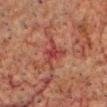| key | value |
|---|---|
| notes | no biopsy performed (imaged during a skin exam) |
| site | the head or neck |
| patient | male, aged approximately 60 |
| acquisition | 15 mm crop, total-body photography |
| TBP lesion metrics | border irregularity of about 5 on a 0–10 scale and radial color variation of about 0.5 |